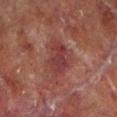biopsy status = imaged on a skin check; not biopsied | image = ~15 mm crop, total-body skin-cancer survey | location = the left lower leg | subject = male, aged approximately 70.